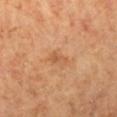Clinical impression:
The lesion was tiled from a total-body skin photograph and was not biopsied.
Acquisition and patient details:
A 15 mm close-up tile from a total-body photography series done for melanoma screening. The total-body-photography lesion software estimated border irregularity of about 3 on a 0–10 scale, a color-variation rating of about 0.5/10, and peripheral color asymmetry of about 0. A female subject, in their 70s. On the right lower leg. The tile uses cross-polarized illumination.